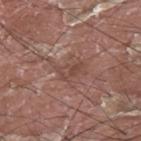Assessment:
No biopsy was performed on this lesion — it was imaged during a full skin examination and was not determined to be concerning.
Background:
A 15 mm close-up extracted from a 3D total-body photography capture. The recorded lesion diameter is about 3 mm. A male patient, about 40 years old. The lesion is located on the back.